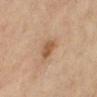Findings:
– biopsy status — catalogued during a skin exam; not biopsied
– subject — female, aged 63 to 67
– body site — the right lower leg
– image source — ~15 mm crop, total-body skin-cancer survey
– tile lighting — cross-polarized